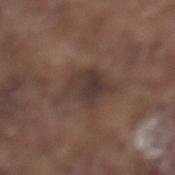A male subject in their 80s. About 5.5 mm across. A close-up tile cropped from a whole-body skin photograph, about 15 mm across. From the left lower leg. The tile uses white-light illumination. The lesion-visualizer software estimated about 8 CIELAB-L* units darker than the surrounding skin and a lesion-to-skin contrast of about 8 (normalized; higher = more distinct). It also reported a within-lesion color-variation index near 4/10.